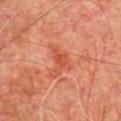• notes — imaged on a skin check; not biopsied
• automated metrics — a lesion area of about 5 mm², an eccentricity of roughly 0.8, and a symmetry-axis asymmetry near 0.45; border irregularity of about 5.5 on a 0–10 scale, internal color variation of about 1.5 on a 0–10 scale, and a peripheral color-asymmetry measure near 0.5
• body site — the chest
• image source — total-body-photography crop, ~15 mm field of view
• lesion size — ≈3.5 mm
• illumination — cross-polarized illumination
• patient — male, aged 73 to 77